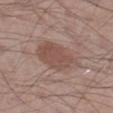Clinical impression:
No biopsy was performed on this lesion — it was imaged during a full skin examination and was not determined to be concerning.
Image and clinical context:
A male patient in their mid- to late 50s. The tile uses white-light illumination. A lesion tile, about 15 mm wide, cut from a 3D total-body photograph. From the right lower leg.